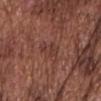Impression:
The lesion was photographed on a routine skin check and not biopsied; there is no pathology result.
Background:
The lesion's longest dimension is about 3 mm. On the front of the torso. The tile uses white-light illumination. A male subject, aged approximately 75. A close-up tile cropped from a whole-body skin photograph, about 15 mm across. The lesion-visualizer software estimated an automated nevus-likeness rating near 0 out of 100.